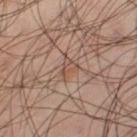Part of a total-body skin-imaging series; this lesion was reviewed on a skin check and was not flagged for biopsy. A male subject, in their 40s. Captured under cross-polarized illumination. A 15 mm close-up extracted from a 3D total-body photography capture. From the leg.